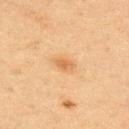Impression: The lesion was photographed on a routine skin check and not biopsied; there is no pathology result. Clinical summary: A male patient about 65 years old. The lesion's longest dimension is about 2.5 mm. From the chest. The tile uses cross-polarized illumination. This image is a 15 mm lesion crop taken from a total-body photograph. Automated tile analysis of the lesion measured an area of roughly 3 mm², an outline eccentricity of about 0.8 (0 = round, 1 = elongated), and a symmetry-axis asymmetry near 0.25. It also reported a border-irregularity rating of about 2/10, a color-variation rating of about 1/10, and radial color variation of about 0.5. The analysis additionally found lesion-presence confidence of about 100/100.